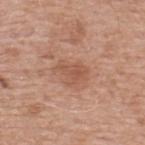Q: Was this lesion biopsied?
A: catalogued during a skin exam; not biopsied
Q: Automated lesion metrics?
A: an area of roughly 7 mm², an outline eccentricity of about 0.75 (0 = round, 1 = elongated), and a symmetry-axis asymmetry near 0.45; a border-irregularity rating of about 4.5/10, a within-lesion color-variation index near 2.5/10, and a peripheral color-asymmetry measure near 1
Q: How was the tile lit?
A: white-light illumination
Q: Where on the body is the lesion?
A: the upper back
Q: What are the patient's age and sex?
A: male, approximately 55 years of age
Q: What is the lesion's diameter?
A: ~4 mm (longest diameter)
Q: How was this image acquired?
A: ~15 mm crop, total-body skin-cancer survey